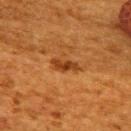{"biopsy_status": "not biopsied; imaged during a skin examination", "image": {"source": "total-body photography crop", "field_of_view_mm": 15}, "site": "upper back", "lighting": "cross-polarized", "lesion_size": {"long_diameter_mm_approx": 3.5}, "patient": {"sex": "female", "age_approx": 50}, "automated_metrics": {"area_mm2_approx": 4.5, "eccentricity": 0.9, "shape_asymmetry": 0.3}}